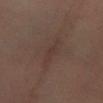Assessment: This lesion was catalogued during total-body skin photography and was not selected for biopsy. Clinical summary: On the left forearm. The tile uses cross-polarized illumination. Measured at roughly 4 mm in maximum diameter. A region of skin cropped from a whole-body photographic capture, roughly 15 mm wide. The subject is a female aged 53–57.